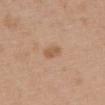The lesion is on the upper back. A 15 mm close-up tile from a total-body photography series done for melanoma screening. The subject is a female aged around 60. Automated image analysis of the tile measured a shape eccentricity near 0.85 and a shape-asymmetry score of about 0.25 (0 = symmetric). The lesion's longest dimension is about 2.5 mm.